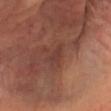The lesion was photographed on a routine skin check and not biopsied; there is no pathology result. A close-up tile cropped from a whole-body skin photograph, about 15 mm across. The subject is a male aged 63 to 67. Captured under cross-polarized illumination. The lesion's longest dimension is about 3 mm. The lesion is on the head or neck.